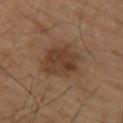workup — total-body-photography surveillance lesion; no biopsy
illumination — cross-polarized
subject — male, aged 53–57
anatomic site — the right forearm
acquisition — total-body-photography crop, ~15 mm field of view
lesion diameter — ~5 mm (longest diameter)
automated lesion analysis — an average lesion color of about L≈39 a*≈18 b*≈28 (CIELAB), about 8 CIELAB-L* units darker than the surrounding skin, and a lesion-to-skin contrast of about 7 (normalized; higher = more distinct); an automated nevus-likeness rating near 65 out of 100 and a detector confidence of about 100 out of 100 that the crop contains a lesion